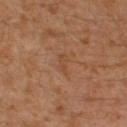Q: Was a biopsy performed?
A: total-body-photography surveillance lesion; no biopsy
Q: Patient demographics?
A: male, aged approximately 30
Q: What is the imaging modality?
A: ~15 mm crop, total-body skin-cancer survey
Q: How was the tile lit?
A: cross-polarized illumination
Q: What is the anatomic site?
A: the left leg